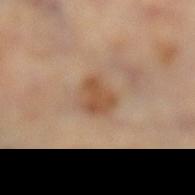anatomic site: the left lower leg; acquisition: ~15 mm crop, total-body skin-cancer survey; lesion diameter: about 3 mm; subject: female, about 60 years old.